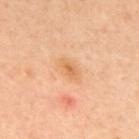{"biopsy_status": "not biopsied; imaged during a skin examination"}